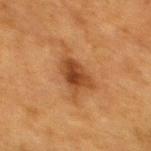Assessment:
Part of a total-body skin-imaging series; this lesion was reviewed on a skin check and was not flagged for biopsy.
Context:
Captured under cross-polarized illumination. From the back. Approximately 4 mm at its widest. The patient is a female approximately 55 years of age. The lesion-visualizer software estimated a lesion area of about 7.5 mm² and an outline eccentricity of about 0.75 (0 = round, 1 = elongated). It also reported a lesion color around L≈36 a*≈22 b*≈33 in CIELAB, a lesion–skin lightness drop of about 10, and a normalized border contrast of about 9. A roughly 15 mm field-of-view crop from a total-body skin photograph.